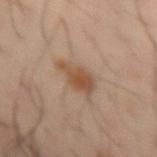workup = total-body-photography surveillance lesion; no biopsy
body site = the left forearm
patient = male, approximately 40 years of age
TBP lesion metrics = a classifier nevus-likeness of about 80/100 and a lesion-detection confidence of about 100/100
imaging modality = ~15 mm tile from a whole-body skin photo